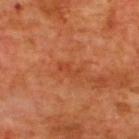Q: Was a biopsy performed?
A: total-body-photography surveillance lesion; no biopsy
Q: Where on the body is the lesion?
A: the upper back
Q: Who is the patient?
A: male, in their mid-60s
Q: How was this image acquired?
A: ~15 mm tile from a whole-body skin photo